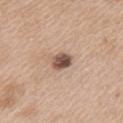| field | value |
|---|---|
| tile lighting | white-light illumination |
| lesion size | about 2.5 mm |
| location | the left upper arm |
| subject | female, approximately 40 years of age |
| imaging modality | total-body-photography crop, ~15 mm field of view |
| TBP lesion metrics | an area of roughly 5 mm², an eccentricity of roughly 0.4, and a symmetry-axis asymmetry near 0.15; a mean CIELAB color near L≈52 a*≈18 b*≈26, roughly 16 lightness units darker than nearby skin, and a lesion-to-skin contrast of about 10.5 (normalized; higher = more distinct); a border-irregularity index near 1/10, a within-lesion color-variation index near 6.5/10, and peripheral color asymmetry of about 2.5 |